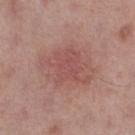Impression: Recorded during total-body skin imaging; not selected for excision or biopsy. Context: Cropped from a total-body skin-imaging series; the visible field is about 15 mm. From the right thigh. A male subject, aged approximately 70.